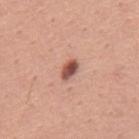Impression: Imaged during a routine full-body skin examination; the lesion was not biopsied and no histopathology is available. Acquisition and patient details: Located on the mid back. This is a white-light tile. A male subject, approximately 45 years of age. Longest diameter approximately 2.5 mm. A 15 mm crop from a total-body photograph taken for skin-cancer surveillance.